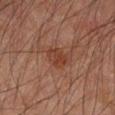Q: What is the anatomic site?
A: the left forearm
Q: What kind of image is this?
A: total-body-photography crop, ~15 mm field of view
Q: What lighting was used for the tile?
A: cross-polarized
Q: Patient demographics?
A: male, approximately 45 years of age
Q: Lesion size?
A: ≈3 mm
Q: What did automated image analysis measure?
A: an outline eccentricity of about 0.7 (0 = round, 1 = elongated) and two-axis asymmetry of about 0.25; a mean CIELAB color near L≈34 a*≈21 b*≈26, roughly 6 lightness units darker than nearby skin, and a lesion-to-skin contrast of about 6.5 (normalized; higher = more distinct); a detector confidence of about 100 out of 100 that the crop contains a lesion